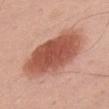Captured under white-light illumination. Automated tile analysis of the lesion measured a footprint of about 35 mm², a shape eccentricity near 0.8, and two-axis asymmetry of about 0.15. The software also gave a mean CIELAB color near L≈54 a*≈26 b*≈30. And it measured a border-irregularity rating of about 2/10, a within-lesion color-variation index near 5/10, and radial color variation of about 1.5. It also reported an automated nevus-likeness rating near 100 out of 100 and lesion-presence confidence of about 100/100. Located on the front of the torso. The patient is a male roughly 55 years of age. This image is a 15 mm lesion crop taken from a total-body photograph. Approximately 8.5 mm at its widest.